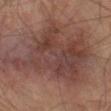Captured during whole-body skin photography for melanoma surveillance; the lesion was not biopsied. A 15 mm crop from a total-body photograph taken for skin-cancer surveillance. A male subject, in their mid- to late 60s. Captured under cross-polarized illumination. On the left thigh.